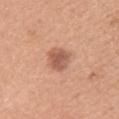Notes:
- notes — total-body-photography surveillance lesion; no biopsy
- acquisition — 15 mm crop, total-body photography
- subject — female, in their 30s
- site — the upper back